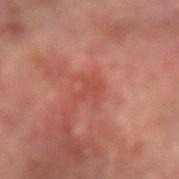Q: Patient demographics?
A: male, about 65 years old
Q: Lesion size?
A: about 2.5 mm
Q: How was the tile lit?
A: cross-polarized illumination
Q: How was this image acquired?
A: 15 mm crop, total-body photography
Q: Lesion location?
A: the arm